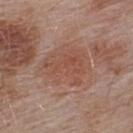Clinical impression: The lesion was tiled from a total-body skin photograph and was not biopsied. Background: The lesion's longest dimension is about 6.5 mm. Imaged with white-light lighting. Automated tile analysis of the lesion measured a lesion area of about 25 mm² and two-axis asymmetry of about 0.3. And it measured an average lesion color of about L≈51 a*≈21 b*≈27 (CIELAB) and a lesion–skin lightness drop of about 6. And it measured a within-lesion color-variation index near 3.5/10 and a peripheral color-asymmetry measure near 1. The software also gave an automated nevus-likeness rating near 0 out of 100 and lesion-presence confidence of about 80/100. The lesion is on the upper back. A male patient, aged approximately 55. A lesion tile, about 15 mm wide, cut from a 3D total-body photograph.